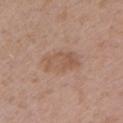Clinical impression: This lesion was catalogued during total-body skin photography and was not selected for biopsy. Context: Cropped from a whole-body photographic skin survey; the tile spans about 15 mm. The lesion is located on the left upper arm. A male subject approximately 50 years of age. The recorded lesion diameter is about 5 mm. This is a white-light tile.